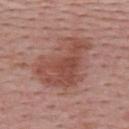| field | value |
|---|---|
| anatomic site | the upper back |
| patient | female, in their mid-50s |
| image | 15 mm crop, total-body photography |
| image-analysis metrics | a lesion area of about 28 mm² and a shape-asymmetry score of about 0.35 (0 = symmetric) |